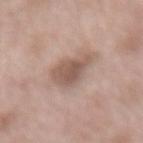The patient is a male about 55 years old.
The lesion is located on the lower back.
A 15 mm close-up tile from a total-body photography series done for melanoma screening.
The recorded lesion diameter is about 4 mm.